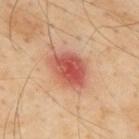{"biopsy_status": "not biopsied; imaged during a skin examination", "patient": {"sex": "male", "age_approx": 55}, "lesion_size": {"long_diameter_mm_approx": 5.0}, "site": "upper back", "image": {"source": "total-body photography crop", "field_of_view_mm": 15}}